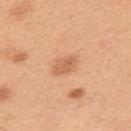A region of skin cropped from a whole-body photographic capture, roughly 15 mm wide.
This is a white-light tile.
Measured at roughly 3 mm in maximum diameter.
An algorithmic analysis of the crop reported an average lesion color of about L≈62 a*≈25 b*≈37 (CIELAB), about 9 CIELAB-L* units darker than the surrounding skin, and a normalized border contrast of about 6. It also reported an automated nevus-likeness rating near 55 out of 100 and a lesion-detection confidence of about 100/100.
A male subject approximately 50 years of age.
Located on the upper back.